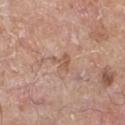Impression: Imaged during a routine full-body skin examination; the lesion was not biopsied and no histopathology is available. Acquisition and patient details: Located on the left lower leg. Cropped from a total-body skin-imaging series; the visible field is about 15 mm. This is a white-light tile. A male subject roughly 80 years of age. Measured at roughly 2.5 mm in maximum diameter.